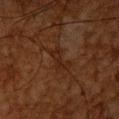Assessment:
The lesion was tiled from a total-body skin photograph and was not biopsied.
Background:
Cropped from a whole-body photographic skin survey; the tile spans about 15 mm. The lesion is located on the upper back. The recorded lesion diameter is about 4 mm. Captured under cross-polarized illumination. Automated image analysis of the tile measured a mean CIELAB color near L≈19 a*≈17 b*≈23, a lesion–skin lightness drop of about 5, and a normalized border contrast of about 6.5. The analysis additionally found border irregularity of about 6 on a 0–10 scale, a color-variation rating of about 2/10, and a peripheral color-asymmetry measure near 0.5. The analysis additionally found an automated nevus-likeness rating near 5 out of 100 and lesion-presence confidence of about 70/100. A male patient aged 63 to 67.